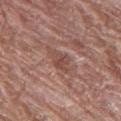Assessment: No biopsy was performed on this lesion — it was imaged during a full skin examination and was not determined to be concerning. Background: A female subject roughly 80 years of age. The lesion's longest dimension is about 3.5 mm. The lesion is on the leg. A 15 mm crop from a total-body photograph taken for skin-cancer surveillance. Imaged with white-light lighting.